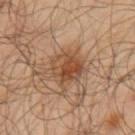Captured during whole-body skin photography for melanoma surveillance; the lesion was not biopsied.
A male patient approximately 65 years of age.
A 15 mm close-up tile from a total-body photography series done for melanoma screening.
The lesion is located on the left upper arm.
The tile uses cross-polarized illumination.
Approximately 4 mm at its widest.
The total-body-photography lesion software estimated a footprint of about 11 mm² and a shape eccentricity near 0.5. And it measured a border-irregularity index near 2.5/10, a within-lesion color-variation index near 7/10, and peripheral color asymmetry of about 2.5. And it measured a nevus-likeness score of about 70/100.